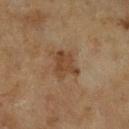biopsy status = catalogued during a skin exam; not biopsied | subject = male, in their mid-60s | diameter = ~3.5 mm (longest diameter) | imaging modality = 15 mm crop, total-body photography | anatomic site = the leg.